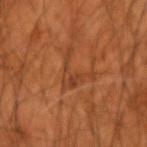Q: Was this lesion biopsied?
A: catalogued during a skin exam; not biopsied
Q: Patient demographics?
A: male, roughly 55 years of age
Q: How was the tile lit?
A: cross-polarized
Q: How was this image acquired?
A: 15 mm crop, total-body photography
Q: How large is the lesion?
A: about 4.5 mm
Q: Lesion location?
A: the right forearm